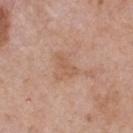• biopsy status · imaged on a skin check; not biopsied
• subject · male, approximately 55 years of age
• image · total-body-photography crop, ~15 mm field of view
• lighting · white-light
• diameter · ~3 mm (longest diameter)
• body site · the back